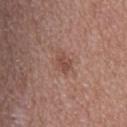Clinical summary: The patient is a female aged approximately 40. The tile uses white-light illumination. Located on the back. Approximately 3 mm at its widest. A roughly 15 mm field-of-view crop from a total-body skin photograph.